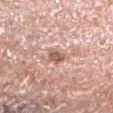Q: Was a biopsy performed?
A: total-body-photography surveillance lesion; no biopsy
Q: How was this image acquired?
A: ~15 mm crop, total-body skin-cancer survey
Q: Lesion location?
A: the left lower leg
Q: Who is the patient?
A: male, in their mid-50s
Q: How large is the lesion?
A: ≈2.5 mm
Q: What lighting was used for the tile?
A: white-light